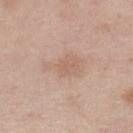Assessment:
The lesion was photographed on a routine skin check and not biopsied; there is no pathology result.